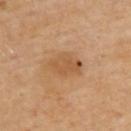Q: Was this lesion biopsied?
A: no biopsy performed (imaged during a skin exam)
Q: What did automated image analysis measure?
A: an area of roughly 8.5 mm², a shape eccentricity near 0.8, and a symmetry-axis asymmetry near 0.25; border irregularity of about 3 on a 0–10 scale and internal color variation of about 2.5 on a 0–10 scale; a classifier nevus-likeness of about 0/100 and lesion-presence confidence of about 100/100
Q: Where on the body is the lesion?
A: the upper back
Q: Patient demographics?
A: male, aged 68 to 72
Q: Lesion size?
A: ~4.5 mm (longest diameter)
Q: How was this image acquired?
A: ~15 mm crop, total-body skin-cancer survey